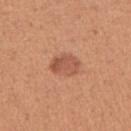This lesion was catalogued during total-body skin photography and was not selected for biopsy. This is a white-light tile. A female subject, about 40 years old. The lesion is located on the left upper arm. A close-up tile cropped from a whole-body skin photograph, about 15 mm across.